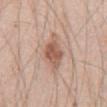follow-up — no biopsy performed (imaged during a skin exam).